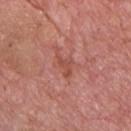Captured during whole-body skin photography for melanoma surveillance; the lesion was not biopsied.
Measured at roughly 3 mm in maximum diameter.
The lesion is on the chest.
A lesion tile, about 15 mm wide, cut from a 3D total-body photograph.
Captured under white-light illumination.
A male patient, in their mid- to late 70s.